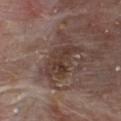Notes:
* biopsy status: imaged on a skin check; not biopsied
* diameter: ≈5 mm
* lighting: white-light illumination
* TBP lesion metrics: about 8 CIELAB-L* units darker than the surrounding skin and a normalized lesion–skin contrast near 7
* subject: male, aged 78–82
* site: the chest
* acquisition: ~15 mm tile from a whole-body skin photo